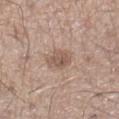<record>
  <biopsy_status>not biopsied; imaged during a skin examination</biopsy_status>
  <site>left lower leg</site>
  <image>
    <source>total-body photography crop</source>
    <field_of_view_mm>15</field_of_view_mm>
  </image>
  <patient>
    <sex>male</sex>
    <age_approx>60</age_approx>
  </patient>
  <lesion_size>
    <long_diameter_mm_approx>3.0</long_diameter_mm_approx>
  </lesion_size>
  <automated_metrics>
    <eccentricity>0.45</eccentricity>
    <shape_asymmetry>0.2</shape_asymmetry>
    <vs_skin_darker_L>9.0</vs_skin_darker_L>
    <vs_skin_contrast_norm>6.5</vs_skin_contrast_norm>
  </automated_metrics>
  <lighting>white-light</lighting>
</record>